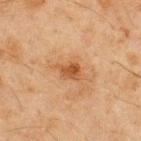imaging modality — ~15 mm crop, total-body skin-cancer survey | subject — male, about 45 years old | tile lighting — cross-polarized illumination | automated lesion analysis — an area of roughly 4 mm², an eccentricity of roughly 0.75, and a shape-asymmetry score of about 0.3 (0 = symmetric); an average lesion color of about L≈43 a*≈21 b*≈35 (CIELAB), a lesion–skin lightness drop of about 9, and a lesion-to-skin contrast of about 8 (normalized; higher = more distinct); a classifier nevus-likeness of about 40/100 | anatomic site — the upper back.